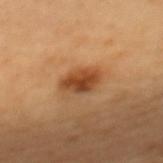workup = no biopsy performed (imaged during a skin exam) | image = ~15 mm crop, total-body skin-cancer survey | automated metrics = a footprint of about 7 mm², a shape eccentricity near 0.8, and two-axis asymmetry of about 0.2; about 13 CIELAB-L* units darker than the surrounding skin and a normalized border contrast of about 9.5; a color-variation rating of about 4.5/10 and peripheral color asymmetry of about 1.5; a classifier nevus-likeness of about 95/100 | size = ~4 mm (longest diameter) | subject = female, approximately 60 years of age | location = the back.